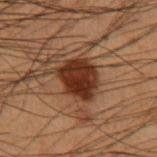Q: Was a biopsy performed?
A: no biopsy performed (imaged during a skin exam)
Q: How large is the lesion?
A: ~4.5 mm (longest diameter)
Q: What is the imaging modality?
A: ~15 mm tile from a whole-body skin photo
Q: How was the tile lit?
A: cross-polarized
Q: Who is the patient?
A: male, roughly 55 years of age
Q: Lesion location?
A: the left upper arm